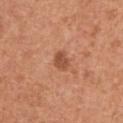No biopsy was performed on this lesion — it was imaged during a full skin examination and was not determined to be concerning. Captured under white-light illumination. Automated tile analysis of the lesion measured an area of roughly 4 mm², a shape eccentricity near 0.7, and a shape-asymmetry score of about 0.25 (0 = symmetric). The analysis additionally found border irregularity of about 2 on a 0–10 scale, a within-lesion color-variation index near 2/10, and radial color variation of about 1. The analysis additionally found an automated nevus-likeness rating near 80 out of 100. The lesion is on the abdomen. Longest diameter approximately 2.5 mm. This image is a 15 mm lesion crop taken from a total-body photograph. A male patient aged 63–67.